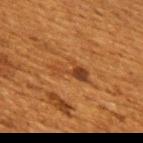<case>
<biopsy_status>not biopsied; imaged during a skin examination</biopsy_status>
<lesion_size>
  <long_diameter_mm_approx>5.0</long_diameter_mm_approx>
</lesion_size>
<image>
  <source>total-body photography crop</source>
  <field_of_view_mm>15</field_of_view_mm>
</image>
<site>upper back</site>
<patient>
  <sex>female</sex>
  <age_approx>50</age_approx>
</patient>
<lighting>cross-polarized</lighting>
</case>